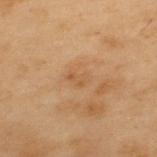| feature | finding |
|---|---|
| workup | imaged on a skin check; not biopsied |
| size | ≈2.5 mm |
| image | ~15 mm crop, total-body skin-cancer survey |
| body site | the upper back |
| subject | male, aged approximately 55 |
| lighting | cross-polarized illumination |